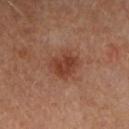Clinical impression: No biopsy was performed on this lesion — it was imaged during a full skin examination and was not determined to be concerning. Clinical summary: The patient is a female roughly 45 years of age. A region of skin cropped from a whole-body photographic capture, roughly 15 mm wide. On the right forearm. Automated tile analysis of the lesion measured a footprint of about 9.5 mm², an eccentricity of roughly 0.55, and two-axis asymmetry of about 0.15. And it measured a classifier nevus-likeness of about 85/100. This is a cross-polarized tile.